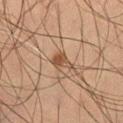workup: imaged on a skin check; not biopsied
lighting: cross-polarized
patient: male, aged 48–52
image source: total-body-photography crop, ~15 mm field of view
automated lesion analysis: an area of roughly 4.5 mm², an outline eccentricity of about 0.8 (0 = round, 1 = elongated), and a shape-asymmetry score of about 0.45 (0 = symmetric); roughly 9 lightness units darker than nearby skin and a normalized lesion–skin contrast near 7.5; a border-irregularity index near 4.5/10 and a within-lesion color-variation index near 2/10
anatomic site: the leg